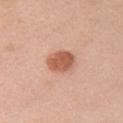follow-up = catalogued during a skin exam; not biopsied | patient = female, about 50 years old | anatomic site = the right upper arm | lesion size = ~3.5 mm (longest diameter) | automated metrics = about 13 CIELAB-L* units darker than the surrounding skin and a normalized lesion–skin contrast near 9; a border-irregularity index near 1.5/10, a color-variation rating of about 2.5/10, and a peripheral color-asymmetry measure near 1; a classifier nevus-likeness of about 100/100 and lesion-presence confidence of about 100/100 | acquisition = ~15 mm crop, total-body skin-cancer survey.